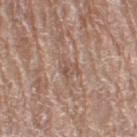<lesion>
<lighting>white-light</lighting>
<image>
  <source>total-body photography crop</source>
  <field_of_view_mm>15</field_of_view_mm>
</image>
<patient>
  <sex>female</sex>
  <age_approx>70</age_approx>
</patient>
<site>leg</site>
</lesion>